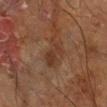notes: no biopsy performed (imaged during a skin exam); image-analysis metrics: a within-lesion color-variation index near 2.5/10 and radial color variation of about 1; subject: male, aged 58–62; body site: the leg; diameter: ~4 mm (longest diameter); acquisition: ~15 mm crop, total-body skin-cancer survey.